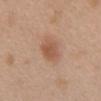Assessment: Imaged during a routine full-body skin examination; the lesion was not biopsied and no histopathology is available. Acquisition and patient details: A female patient aged 23 to 27. A lesion tile, about 15 mm wide, cut from a 3D total-body photograph. The lesion is on the chest.